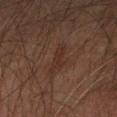The lesion was photographed on a routine skin check and not biopsied; there is no pathology result.
Imaged with cross-polarized lighting.
The recorded lesion diameter is about 4 mm.
A male subject approximately 65 years of age.
An algorithmic analysis of the crop reported an area of roughly 5 mm², an eccentricity of roughly 0.95, and two-axis asymmetry of about 0.35. The software also gave an automated nevus-likeness rating near 0 out of 100 and a detector confidence of about 90 out of 100 that the crop contains a lesion.
A lesion tile, about 15 mm wide, cut from a 3D total-body photograph.
On the right forearm.